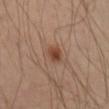• workup — total-body-photography surveillance lesion; no biopsy
• acquisition — ~15 mm tile from a whole-body skin photo
• site — the left upper arm
• patient — male, roughly 65 years of age